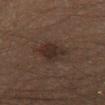No biopsy was performed on this lesion — it was imaged during a full skin examination and was not determined to be concerning.
A male patient approximately 60 years of age.
Automated image analysis of the tile measured a border-irregularity rating of about 2.5/10, internal color variation of about 3 on a 0–10 scale, and peripheral color asymmetry of about 1. It also reported a nevus-likeness score of about 10/100 and a lesion-detection confidence of about 100/100.
The tile uses cross-polarized illumination.
Cropped from a whole-body photographic skin survey; the tile spans about 15 mm.
On the leg.
The lesion's longest dimension is about 4 mm.